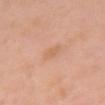| field | value |
|---|---|
| notes | catalogued during a skin exam; not biopsied |
| anatomic site | the front of the torso |
| TBP lesion metrics | a border-irregularity rating of about 3.5/10 and a within-lesion color-variation index near 0.5/10; a classifier nevus-likeness of about 0/100 |
| illumination | white-light |
| image source | ~15 mm crop, total-body skin-cancer survey |
| subject | female, approximately 50 years of age |